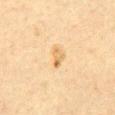Notes:
• lesion diameter: ≈2.5 mm
• body site: the abdomen
• imaging modality: ~15 mm crop, total-body skin-cancer survey
• patient: male, aged 58–62